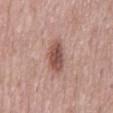{"biopsy_status": "not biopsied; imaged during a skin examination", "image": {"source": "total-body photography crop", "field_of_view_mm": 15}, "automated_metrics": {"area_mm2_approx": 8.5, "eccentricity": 0.9, "cielab_L": 52, "cielab_a": 21, "cielab_b": 24, "nevus_likeness_0_100": 95}, "patient": {"sex": "male", "age_approx": 55}, "lighting": "white-light", "site": "mid back", "lesion_size": {"long_diameter_mm_approx": 5.0}}